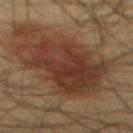  biopsy_status: not biopsied; imaged during a skin examination
  lesion_size:
    long_diameter_mm_approx: 8.5
  patient:
    sex: male
    age_approx: 60
  site: abdomen
  image:
    source: total-body photography crop
    field_of_view_mm: 15
  automated_metrics:
    area_mm2_approx: 42.0
    eccentricity: 0.7
    shape_asymmetry: 0.25
    cielab_L: 32
    cielab_a: 18
    cielab_b: 25
    vs_skin_contrast_norm: 8.5
    border_irregularity_0_10: 4.5
    color_variation_0_10: 4.5
    peripheral_color_asymmetry: 1.5